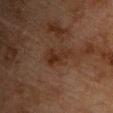Q: Was this lesion biopsied?
A: catalogued during a skin exam; not biopsied
Q: Where on the body is the lesion?
A: the chest
Q: Patient demographics?
A: male, aged approximately 75
Q: What kind of image is this?
A: ~15 mm crop, total-body skin-cancer survey